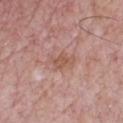The lesion was photographed on a routine skin check and not biopsied; there is no pathology result.
A close-up tile cropped from a whole-body skin photograph, about 15 mm across.
A male subject in their mid-50s.